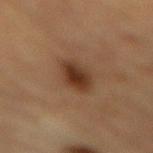No biopsy was performed on this lesion — it was imaged during a full skin examination and was not determined to be concerning.
The lesion is located on the mid back.
This is a cross-polarized tile.
Longest diameter approximately 4 mm.
A region of skin cropped from a whole-body photographic capture, roughly 15 mm wide.
The subject is a male roughly 85 years of age or older.